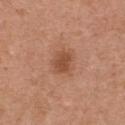<case>
  <biopsy_status>not biopsied; imaged during a skin examination</biopsy_status>
  <lighting>white-light</lighting>
  <lesion_size>
    <long_diameter_mm_approx>3.0</long_diameter_mm_approx>
  </lesion_size>
  <automated_metrics>
    <cielab_L>49</cielab_L>
    <cielab_a>24</cielab_a>
    <cielab_b>33</cielab_b>
    <vs_skin_darker_L>10.0</vs_skin_darker_L>
    <border_irregularity_0_10>2.0</border_irregularity_0_10>
    <color_variation_0_10>1.5</color_variation_0_10>
    <nevus_likeness_0_100>50</nevus_likeness_0_100>
    <lesion_detection_confidence_0_100>100</lesion_detection_confidence_0_100>
  </automated_metrics>
  <image>
    <source>total-body photography crop</source>
    <field_of_view_mm>15</field_of_view_mm>
  </image>
  <site>chest</site>
  <patient>
    <sex>female</sex>
    <age_approx>30</age_approx>
  </patient>
</case>